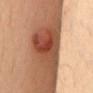biopsy status: total-body-photography surveillance lesion; no biopsy
lighting: cross-polarized illumination
anatomic site: the mid back
acquisition: 15 mm crop, total-body photography
diameter: ~6 mm (longest diameter)
subject: female, aged approximately 35